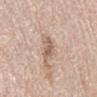No biopsy was performed on this lesion — it was imaged during a full skin examination and was not determined to be concerning. The subject is a female aged 58–62. Automated tile analysis of the lesion measured an area of roughly 5.5 mm², a shape eccentricity near 0.85, and a shape-asymmetry score of about 0.4 (0 = symmetric). The software also gave a detector confidence of about 95 out of 100 that the crop contains a lesion. The recorded lesion diameter is about 3.5 mm. Located on the abdomen. This is a white-light tile. A region of skin cropped from a whole-body photographic capture, roughly 15 mm wide.